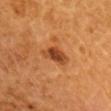• notes: total-body-photography surveillance lesion; no biopsy
• TBP lesion metrics: a footprint of about 6.5 mm², an eccentricity of roughly 0.85, and a shape-asymmetry score of about 0.35 (0 = symmetric); a classifier nevus-likeness of about 95/100 and a detector confidence of about 100 out of 100 that the crop contains a lesion
• imaging modality: total-body-photography crop, ~15 mm field of view
• tile lighting: cross-polarized illumination
• patient: approximately 70 years of age
• site: the head or neck
• diameter: ≈4 mm